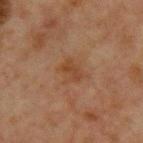<lesion>
  <biopsy_status>not biopsied; imaged during a skin examination</biopsy_status>
  <patient>
    <sex>male</sex>
    <age_approx>65</age_approx>
  </patient>
  <image>
    <source>total-body photography crop</source>
    <field_of_view_mm>15</field_of_view_mm>
  </image>
  <site>front of the torso</site>
  <lighting>cross-polarized</lighting>
</lesion>